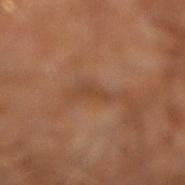notes: total-body-photography surveillance lesion; no biopsy
patient: male, aged approximately 60
image source: ~15 mm tile from a whole-body skin photo
automated metrics: an area of roughly 2.5 mm², an eccentricity of roughly 0.9, and a shape-asymmetry score of about 0.5 (0 = symmetric); a lesion color around L≈41 a*≈21 b*≈31 in CIELAB, about 6 CIELAB-L* units darker than the surrounding skin, and a normalized border contrast of about 5; a border-irregularity rating of about 5/10, internal color variation of about 0 on a 0–10 scale, and a peripheral color-asymmetry measure near 0; an automated nevus-likeness rating near 0 out of 100 and a detector confidence of about 65 out of 100 that the crop contains a lesion
lighting: cross-polarized
anatomic site: the right leg
lesion diameter: ~2.5 mm (longest diameter)